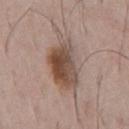This lesion was catalogued during total-body skin photography and was not selected for biopsy. The subject is a male in their mid- to late 60s. A lesion tile, about 15 mm wide, cut from a 3D total-body photograph. The recorded lesion diameter is about 7 mm. From the chest. Imaged with white-light lighting.